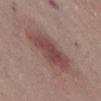workup — total-body-photography surveillance lesion; no biopsy | location — the abdomen | imaging modality — total-body-photography crop, ~15 mm field of view | subject — male, aged approximately 75 | image-analysis metrics — a mean CIELAB color near L≈46 a*≈22 b*≈20, roughly 10 lightness units darker than nearby skin, and a lesion-to-skin contrast of about 7.5 (normalized; higher = more distinct); a classifier nevus-likeness of about 80/100 | tile lighting — white-light illumination | diameter — ~6.5 mm (longest diameter).